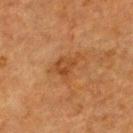Impression: Part of a total-body skin-imaging series; this lesion was reviewed on a skin check and was not flagged for biopsy. Image and clinical context: A female patient approximately 55 years of age. A region of skin cropped from a whole-body photographic capture, roughly 15 mm wide. Approximately 3.5 mm at its widest. Located on the upper back. This is a cross-polarized tile.